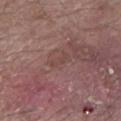Notes:
- biopsy status — no biopsy performed (imaged during a skin exam)
- imaging modality — ~15 mm tile from a whole-body skin photo
- body site — the leg
- illumination — white-light illumination
- lesion size — ~2.5 mm (longest diameter)
- automated metrics — an area of roughly 2.5 mm² and a symmetry-axis asymmetry near 0.4; border irregularity of about 4.5 on a 0–10 scale
- subject — male, about 80 years old